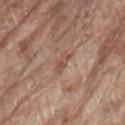Clinical impression:
The lesion was photographed on a routine skin check and not biopsied; there is no pathology result.
Context:
A roughly 15 mm field-of-view crop from a total-body skin photograph. Imaged with white-light lighting. On the lower back. Approximately 3 mm at its widest. The subject is a male aged approximately 80.